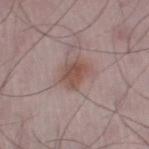The tile uses white-light illumination. The lesion is on the left thigh. A male subject, roughly 50 years of age. Longest diameter approximately 3 mm. A 15 mm crop from a total-body photograph taken for skin-cancer surveillance.